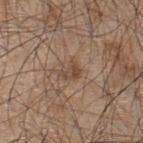The lesion was photographed on a routine skin check and not biopsied; there is no pathology result.
This image is a 15 mm lesion crop taken from a total-body photograph.
Imaged with white-light lighting.
About 2.5 mm across.
The lesion-visualizer software estimated an area of roughly 4 mm², an eccentricity of roughly 0.65, and two-axis asymmetry of about 0.35. It also reported an average lesion color of about L≈46 a*≈17 b*≈27 (CIELAB), a lesion–skin lightness drop of about 8, and a normalized border contrast of about 6.5.
A male subject, roughly 45 years of age.
On the upper back.